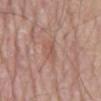workup — catalogued during a skin exam; not biopsied | site — the abdomen | patient — male, aged 78 to 82 | imaging modality — ~15 mm tile from a whole-body skin photo | automated metrics — a color-variation rating of about 2/10; a nevus-likeness score of about 0/100.